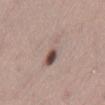Acquisition and patient details: The patient is a male in their mid- to late 40s. The lesion is located on the lower back. Measured at roughly 4.5 mm in maximum diameter. A roughly 15 mm field-of-view crop from a total-body skin photograph. This is a white-light tile.